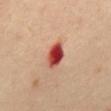{
  "biopsy_status": "not biopsied; imaged during a skin examination",
  "site": "mid back",
  "lighting": "cross-polarized",
  "patient": {
    "sex": "female",
    "age_approx": 55
  },
  "lesion_size": {
    "long_diameter_mm_approx": 3.5
  },
  "image": {
    "source": "total-body photography crop",
    "field_of_view_mm": 15
  }
}